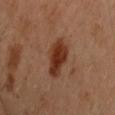<lesion>
  <biopsy_status>not biopsied; imaged during a skin examination</biopsy_status>
  <lighting>cross-polarized</lighting>
  <lesion_size>
    <long_diameter_mm_approx>4.5</long_diameter_mm_approx>
  </lesion_size>
  <site>left upper arm</site>
  <patient>
    <sex>male</sex>
    <age_approx>45</age_approx>
  </patient>
  <automated_metrics>
    <cielab_L>32</cielab_L>
    <cielab_a>22</cielab_a>
    <cielab_b>29</cielab_b>
    <vs_skin_contrast_norm>10.5</vs_skin_contrast_norm>
  </automated_metrics>
  <image>
    <source>total-body photography crop</source>
    <field_of_view_mm>15</field_of_view_mm>
  </image>
</lesion>